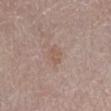| key | value |
|---|---|
| workup | total-body-photography surveillance lesion; no biopsy |
| lighting | white-light illumination |
| diameter | ≈2.5 mm |
| patient | male, aged 83 or older |
| site | the abdomen |
| image | 15 mm crop, total-body photography |